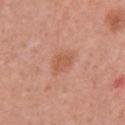notes = no biopsy performed (imaged during a skin exam).